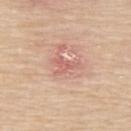- image-analysis metrics · an eccentricity of roughly 0.8 and two-axis asymmetry of about 0.65
- site · the upper back
- subject · female, aged 68–72
- image source · 15 mm crop, total-body photography
- illumination · white-light
- lesion diameter · about 3.5 mm